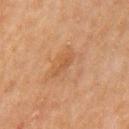<record>
<biopsy_status>not biopsied; imaged during a skin examination</biopsy_status>
<lighting>cross-polarized</lighting>
<patient>
  <sex>male</sex>
  <age_approx>65</age_approx>
</patient>
<lesion_size>
  <long_diameter_mm_approx>3.0</long_diameter_mm_approx>
</lesion_size>
<site>back</site>
<image>
  <source>total-body photography crop</source>
  <field_of_view_mm>15</field_of_view_mm>
</image>
<automated_metrics>
  <cielab_L>47</cielab_L>
  <cielab_a>21</cielab_a>
  <cielab_b>33</cielab_b>
  <vs_skin_contrast_norm>4.5</vs_skin_contrast_norm>
</automated_metrics>
</record>